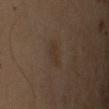Part of a total-body skin-imaging series; this lesion was reviewed on a skin check and was not flagged for biopsy.
The total-body-photography lesion software estimated a lesion area of about 4 mm², an outline eccentricity of about 0.85 (0 = round, 1 = elongated), and a shape-asymmetry score of about 0.35 (0 = symmetric). The analysis additionally found an average lesion color of about L≈31 a*≈12 b*≈23 (CIELAB), roughly 4 lightness units darker than nearby skin, and a normalized border contrast of about 5. And it measured a nevus-likeness score of about 20/100 and a lesion-detection confidence of about 100/100.
Longest diameter approximately 3 mm.
This image is a 15 mm lesion crop taken from a total-body photograph.
Located on the right upper arm.
This is a cross-polarized tile.
A male subject roughly 50 years of age.